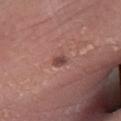Clinical impression:
No biopsy was performed on this lesion — it was imaged during a full skin examination and was not determined to be concerning.
Context:
Cropped from a total-body skin-imaging series; the visible field is about 15 mm. Captured under white-light illumination. The subject is a female roughly 60 years of age. The total-body-photography lesion software estimated a footprint of about 2.5 mm² and two-axis asymmetry of about 0.15. The software also gave a border-irregularity index near 1.5/10 and radial color variation of about 1. The software also gave an automated nevus-likeness rating near 70 out of 100. The lesion is located on the right lower leg.